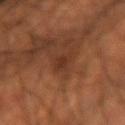Assessment:
This lesion was catalogued during total-body skin photography and was not selected for biopsy.
Acquisition and patient details:
The lesion is located on the left forearm. A lesion tile, about 15 mm wide, cut from a 3D total-body photograph. The subject is a male aged 63–67.